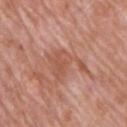A 15 mm close-up tile from a total-body photography series done for melanoma screening. A male subject, aged 68 to 72. On the upper back.